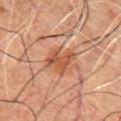{
  "lighting": "cross-polarized",
  "site": "chest",
  "image": {
    "source": "total-body photography crop",
    "field_of_view_mm": 15
  },
  "lesion_size": {
    "long_diameter_mm_approx": 3.5
  },
  "automated_metrics": {
    "nevus_likeness_0_100": 35
  },
  "patient": {
    "sex": "male",
    "age_approx": 50
  }
}